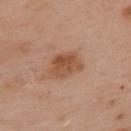The lesion was photographed on a routine skin check and not biopsied; there is no pathology result. Cropped from a whole-body photographic skin survey; the tile spans about 15 mm. The lesion is located on the upper back. A male patient aged 53 to 57.